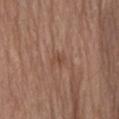From the right forearm. Imaged with white-light lighting. Approximately 1.5 mm at its widest. The subject is a male aged 68 to 72. Cropped from a total-body skin-imaging series; the visible field is about 15 mm. The lesion-visualizer software estimated an area of roughly 2 mm², an outline eccentricity of about 0.6 (0 = round, 1 = elongated), and a symmetry-axis asymmetry near 0.35.